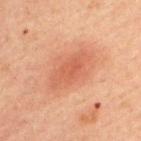  biopsy_status: not biopsied; imaged during a skin examination
  site: upper back
  image:
    source: total-body photography crop
    field_of_view_mm: 15
  patient:
    sex: male
    age_approx: 60
  lesion_size:
    long_diameter_mm_approx: 5.5